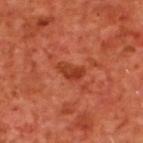Impression:
Recorded during total-body skin imaging; not selected for excision or biopsy.
Clinical summary:
This image is a 15 mm lesion crop taken from a total-body photograph. A male subject, approximately 70 years of age. From the upper back. Longest diameter approximately 3 mm. Captured under cross-polarized illumination.